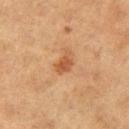The lesion was tiled from a total-body skin photograph and was not biopsied.
A roughly 15 mm field-of-view crop from a total-body skin photograph.
Captured under cross-polarized illumination.
Longest diameter approximately 2.5 mm.
A female patient, aged approximately 40.
On the right thigh.